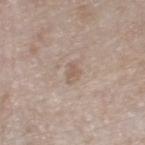Clinical impression:
This lesion was catalogued during total-body skin photography and was not selected for biopsy.
Background:
A 15 mm crop from a total-body photograph taken for skin-cancer surveillance. A female patient, aged 73 to 77. Located on the left thigh.